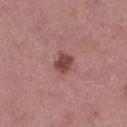Q: Was a biopsy performed?
A: no biopsy performed (imaged during a skin exam)
Q: What is the imaging modality?
A: total-body-photography crop, ~15 mm field of view
Q: Who is the patient?
A: female, aged approximately 40
Q: Lesion location?
A: the leg